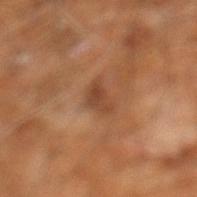Q: Was a biopsy performed?
A: imaged on a skin check; not biopsied
Q: Illumination type?
A: cross-polarized
Q: What are the patient's age and sex?
A: male, aged 58–62
Q: What is the lesion's diameter?
A: ≈3 mm
Q: What is the imaging modality?
A: total-body-photography crop, ~15 mm field of view
Q: What is the anatomic site?
A: the right leg
Q: What did automated image analysis measure?
A: an eccentricity of roughly 0.8 and a symmetry-axis asymmetry near 0.35; a lesion–skin lightness drop of about 8 and a normalized border contrast of about 7; a border-irregularity rating of about 3.5/10, internal color variation of about 2 on a 0–10 scale, and radial color variation of about 0.5; a nevus-likeness score of about 0/100 and lesion-presence confidence of about 100/100